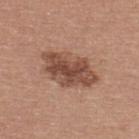workup: catalogued during a skin exam; not biopsied | automated lesion analysis: an area of roughly 18 mm², an eccentricity of roughly 0.85, and a symmetry-axis asymmetry near 0.25; a lesion color around L≈48 a*≈21 b*≈28 in CIELAB, about 13 CIELAB-L* units darker than the surrounding skin, and a normalized border contrast of about 9; border irregularity of about 3 on a 0–10 scale, a within-lesion color-variation index near 5/10, and a peripheral color-asymmetry measure near 2 | site: the back | patient: male, about 40 years old | image: ~15 mm crop, total-body skin-cancer survey | diameter: ≈6.5 mm | tile lighting: white-light illumination.